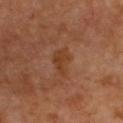Clinical impression: Captured during whole-body skin photography for melanoma surveillance; the lesion was not biopsied. Clinical summary: Longest diameter approximately 4 mm. An algorithmic analysis of the crop reported border irregularity of about 4.5 on a 0–10 scale and internal color variation of about 1.5 on a 0–10 scale. Cropped from a whole-body photographic skin survey; the tile spans about 15 mm. The tile uses cross-polarized illumination. Located on the upper back. A male subject, about 60 years old.